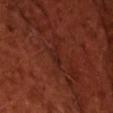Part of a total-body skin-imaging series; this lesion was reviewed on a skin check and was not flagged for biopsy.
A lesion tile, about 15 mm wide, cut from a 3D total-body photograph.
A male subject, aged around 50.
An algorithmic analysis of the crop reported an eccentricity of roughly 0.9 and two-axis asymmetry of about 0.35. The analysis additionally found an average lesion color of about L≈23 a*≈25 b*≈25 (CIELAB) and about 5 CIELAB-L* units darker than the surrounding skin. And it measured a border-irregularity rating of about 4/10 and a color-variation rating of about 0.5/10.
Captured under cross-polarized illumination.
The lesion's longest dimension is about 2.5 mm.
The lesion is located on the head or neck.